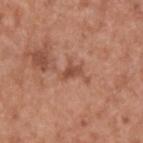Context:
This is a white-light tile. The subject is a male in their mid-60s. Located on the upper back. A lesion tile, about 15 mm wide, cut from a 3D total-body photograph.